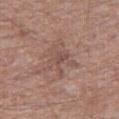workup — catalogued during a skin exam; not biopsied | tile lighting — white-light | automated metrics — a footprint of about 11 mm²; a border-irregularity index near 7.5/10, a within-lesion color-variation index near 3/10, and radial color variation of about 1; a nevus-likeness score of about 0/100 and lesion-presence confidence of about 85/100 | acquisition — ~15 mm crop, total-body skin-cancer survey | patient — male, aged approximately 65 | body site — the right thigh | diameter — ≈5 mm.